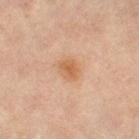No biopsy was performed on this lesion — it was imaged during a full skin examination and was not determined to be concerning. A close-up tile cropped from a whole-body skin photograph, about 15 mm across. The lesion is located on the leg. The subject is a female in their 50s.